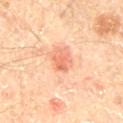{
  "biopsy_status": "not biopsied; imaged during a skin examination"
}